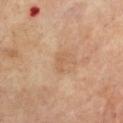Acquisition and patient details: A roughly 15 mm field-of-view crop from a total-body skin photograph. This is a cross-polarized tile. The lesion's longest dimension is about 2.5 mm. A male subject, in their 70s. From the chest.